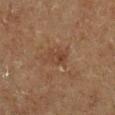The lesion was tiled from a total-body skin photograph and was not biopsied. The patient is a male aged 73–77. This image is a 15 mm lesion crop taken from a total-body photograph. Measured at roughly 2.5 mm in maximum diameter. From the leg. Automated tile analysis of the lesion measured a normalized lesion–skin contrast near 5. The tile uses cross-polarized illumination.